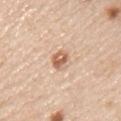site: the chest | image source: ~15 mm crop, total-body skin-cancer survey | patient: male, roughly 45 years of age.